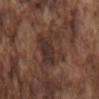Assessment:
Captured during whole-body skin photography for melanoma surveillance; the lesion was not biopsied.
Clinical summary:
A 15 mm crop from a total-body photograph taken for skin-cancer surveillance. The tile uses white-light illumination. A male patient aged around 75. Measured at roughly 5 mm in maximum diameter. An algorithmic analysis of the crop reported an outline eccentricity of about 0.45 (0 = round, 1 = elongated) and a shape-asymmetry score of about 0.3 (0 = symmetric). The software also gave a lesion color around L≈32 a*≈17 b*≈21 in CIELAB. Located on the mid back.